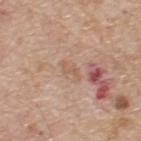Impression: Imaged during a routine full-body skin examination; the lesion was not biopsied and no histopathology is available. Context: The subject is a male aged 68 to 72. A close-up tile cropped from a whole-body skin photograph, about 15 mm across. From the upper back.